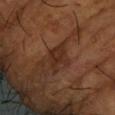follow-up: no biopsy performed (imaged during a skin exam) | anatomic site: the left forearm | lighting: cross-polarized | lesion diameter: ~3.5 mm (longest diameter) | image: ~15 mm tile from a whole-body skin photo | patient: male, aged 63–67.